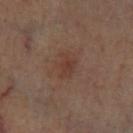{"biopsy_status": "not biopsied; imaged during a skin examination", "image": {"source": "total-body photography crop", "field_of_view_mm": 15}, "lighting": "cross-polarized", "automated_metrics": {"nevus_likeness_0_100": 40, "lesion_detection_confidence_0_100": 100}, "lesion_size": {"long_diameter_mm_approx": 3.0}, "site": "left lower leg"}